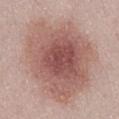Cropped from a whole-body photographic skin survey; the tile spans about 15 mm. Located on the abdomen. This is a white-light tile. Measured at roughly 10 mm in maximum diameter. A female subject in their 50s. The lesion-visualizer software estimated a footprint of about 65 mm² and a shape-asymmetry score of about 0.2 (0 = symmetric). The software also gave roughly 12 lightness units darker than nearby skin and a lesion-to-skin contrast of about 8 (normalized; higher = more distinct). And it measured a within-lesion color-variation index near 6/10 and radial color variation of about 1.5. And it measured an automated nevus-likeness rating near 95 out of 100 and a detector confidence of about 100 out of 100 that the crop contains a lesion.